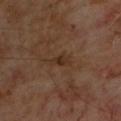Impression: The lesion was tiled from a total-body skin photograph and was not biopsied. Image and clinical context: The patient is a male in their 70s. The lesion is located on the upper back. Captured under cross-polarized illumination. A 15 mm close-up tile from a total-body photography series done for melanoma screening.